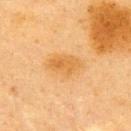Clinical impression: No biopsy was performed on this lesion — it was imaged during a full skin examination and was not determined to be concerning. Clinical summary: The total-body-photography lesion software estimated a mean CIELAB color near L≈56 a*≈20 b*≈43, a lesion–skin lightness drop of about 7, and a normalized border contrast of about 6.5. The software also gave a border-irregularity rating of about 2.5/10, a within-lesion color-variation index near 3/10, and a peripheral color-asymmetry measure near 1. The lesion is located on the back. Cropped from a whole-body photographic skin survey; the tile spans about 15 mm. The lesion's longest dimension is about 4 mm. Imaged with cross-polarized lighting. A female subject about 40 years old.